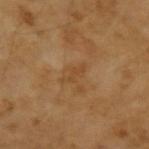Case summary:
* follow-up · no biopsy performed (imaged during a skin exam)
* lighting · cross-polarized illumination
* diameter · ≈3 mm
* patient · male, aged 43–47
* site · the left forearm
* acquisition · ~15 mm tile from a whole-body skin photo